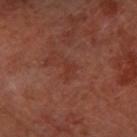<lesion>
  <biopsy_status>not biopsied; imaged during a skin examination</biopsy_status>
  <patient>
    <sex>female</sex>
    <age_approx>65</age_approx>
  </patient>
  <image>
    <source>total-body photography crop</source>
    <field_of_view_mm>15</field_of_view_mm>
  </image>
  <site>left forearm</site>
</lesion>